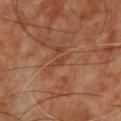Clinical impression: This lesion was catalogued during total-body skin photography and was not selected for biopsy. Background: A male subject about 60 years old. This is a cross-polarized tile. On the chest. This image is a 15 mm lesion crop taken from a total-body photograph. Measured at roughly 2.5 mm in maximum diameter.